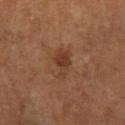  biopsy_status: not biopsied; imaged during a skin examination
  site: right lower leg
  lighting: cross-polarized
  automated_metrics:
    area_mm2_approx: 6.0
    eccentricity: 0.85
    shape_asymmetry: 0.35
    cielab_L: 37
    cielab_a: 20
    cielab_b: 30
    vs_skin_darker_L: 7.0
    vs_skin_contrast_norm: 6.5
    nevus_likeness_0_100: 60
    lesion_detection_confidence_0_100: 100
  image:
    source: total-body photography crop
    field_of_view_mm: 15
  patient:
    sex: female
    age_approx: 65